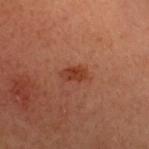Q: Is there a histopathology result?
A: total-body-photography surveillance lesion; no biopsy
Q: What lighting was used for the tile?
A: cross-polarized
Q: What kind of image is this?
A: ~15 mm tile from a whole-body skin photo
Q: What did automated image analysis measure?
A: a mean CIELAB color near L≈34 a*≈24 b*≈29 and a lesion-to-skin contrast of about 7.5 (normalized; higher = more distinct); a border-irregularity index near 2/10 and peripheral color asymmetry of about 1; a classifier nevus-likeness of about 90/100 and a lesion-detection confidence of about 100/100
Q: What is the lesion's diameter?
A: ≈3 mm
Q: What is the anatomic site?
A: the head or neck
Q: What are the patient's age and sex?
A: female, aged around 50